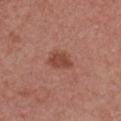<lesion>
  <biopsy_status>not biopsied; imaged during a skin examination</biopsy_status>
  <lesion_size>
    <long_diameter_mm_approx>3.5</long_diameter_mm_approx>
  </lesion_size>
  <image>
    <source>total-body photography crop</source>
    <field_of_view_mm>15</field_of_view_mm>
  </image>
  <patient>
    <sex>female</sex>
    <age_approx>55</age_approx>
  </patient>
  <lighting>white-light</lighting>
  <automated_metrics>
    <nevus_likeness_0_100>80</nevus_likeness_0_100>
    <lesion_detection_confidence_0_100>100</lesion_detection_confidence_0_100>
  </automated_metrics>
  <site>chest</site>
</lesion>